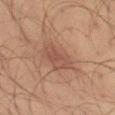| feature | finding |
|---|---|
| workup | no biopsy performed (imaged during a skin exam) |
| lesion size | ~4.5 mm (longest diameter) |
| subject | male, aged approximately 35 |
| image source | ~15 mm crop, total-body skin-cancer survey |
| lighting | cross-polarized |
| site | the leg |
| automated lesion analysis | a footprint of about 9.5 mm², an eccentricity of roughly 0.8, and a symmetry-axis asymmetry near 0.25; a mean CIELAB color near L≈46 a*≈19 b*≈26, a lesion–skin lightness drop of about 7, and a normalized lesion–skin contrast near 6 |